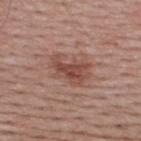{"biopsy_status": "not biopsied; imaged during a skin examination", "automated_metrics": {"area_mm2_approx": 10.0, "eccentricity": 0.75, "shape_asymmetry": 0.3, "color_variation_0_10": 3.5, "nevus_likeness_0_100": 80, "lesion_detection_confidence_0_100": 100}, "image": {"source": "total-body photography crop", "field_of_view_mm": 15}, "lighting": "white-light", "site": "upper back", "lesion_size": {"long_diameter_mm_approx": 4.5}, "patient": {"sex": "male", "age_approx": 40}}